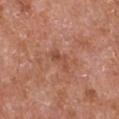Part of a total-body skin-imaging series; this lesion was reviewed on a skin check and was not flagged for biopsy.
This image is a 15 mm lesion crop taken from a total-body photograph.
The lesion is on the left upper arm.
This is a white-light tile.
A male patient, aged around 65.
About 3 mm across.